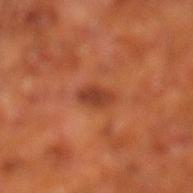Imaged during a routine full-body skin examination; the lesion was not biopsied and no histopathology is available. Located on the left lower leg. A male subject in their mid-60s. A 15 mm crop from a total-body photograph taken for skin-cancer surveillance.